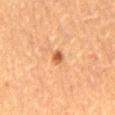notes = total-body-photography surveillance lesion; no biopsy
image-analysis metrics = an area of roughly 2.5 mm², a shape eccentricity near 0.5, and a symmetry-axis asymmetry near 0.15; a border-irregularity rating of about 1.5/10, internal color variation of about 2 on a 0–10 scale, and radial color variation of about 0.5
acquisition = ~15 mm tile from a whole-body skin photo
subject = male, aged approximately 55
site = the mid back
lesion size = ≈2 mm
illumination = cross-polarized